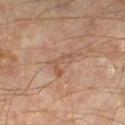Notes:
- follow-up · no biopsy performed (imaged during a skin exam)
- image · ~15 mm crop, total-body skin-cancer survey
- subject · male, aged 58–62
- illumination · cross-polarized
- location · the leg
- TBP lesion metrics · an eccentricity of roughly 0.9 and a symmetry-axis asymmetry near 0.6; a lesion color around L≈50 a*≈18 b*≈29 in CIELAB, a lesion–skin lightness drop of about 6, and a normalized border contrast of about 5; a border-irregularity rating of about 7/10 and internal color variation of about 0 on a 0–10 scale
- size · ~4 mm (longest diameter)